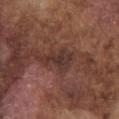The lesion was photographed on a routine skin check and not biopsied; there is no pathology result. The lesion is on the chest. Automated tile analysis of the lesion measured an area of roughly 5.5 mm², an eccentricity of roughly 0.8, and a shape-asymmetry score of about 0.55 (0 = symmetric). It also reported an average lesion color of about L≈33 a*≈19 b*≈21 (CIELAB), about 8 CIELAB-L* units darker than the surrounding skin, and a lesion-to-skin contrast of about 8.5 (normalized; higher = more distinct). The analysis additionally found a border-irregularity rating of about 6/10, a color-variation rating of about 2.5/10, and a peripheral color-asymmetry measure near 1. About 3.5 mm across. This image is a 15 mm lesion crop taken from a total-body photograph. The patient is a male about 75 years old. Imaged with white-light lighting.